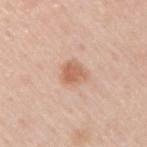Q: Was this lesion biopsied?
A: total-body-photography surveillance lesion; no biopsy
Q: What did automated image analysis measure?
A: a footprint of about 6.5 mm² and two-axis asymmetry of about 0.25
Q: How was this image acquired?
A: ~15 mm crop, total-body skin-cancer survey
Q: What is the anatomic site?
A: the right upper arm
Q: What are the patient's age and sex?
A: male, roughly 60 years of age
Q: What lighting was used for the tile?
A: white-light illumination